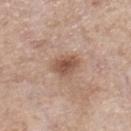Imaged during a routine full-body skin examination; the lesion was not biopsied and no histopathology is available. A 15 mm close-up extracted from a 3D total-body photography capture. An algorithmic analysis of the crop reported an average lesion color of about L≈52 a*≈21 b*≈27 (CIELAB) and a normalized border contrast of about 8. And it measured internal color variation of about 3.5 on a 0–10 scale and radial color variation of about 1. This is a white-light tile. A female patient aged 53–57. Located on the right lower leg. About 3.5 mm across.